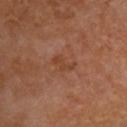Captured during whole-body skin photography for melanoma surveillance; the lesion was not biopsied.
The lesion is located on the upper back.
A female subject approximately 50 years of age.
A 15 mm close-up extracted from a 3D total-body photography capture.